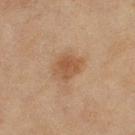Assessment:
The lesion was tiled from a total-body skin photograph and was not biopsied.
Clinical summary:
The patient is a female aged approximately 60. The lesion-visualizer software estimated a footprint of about 8 mm², an outline eccentricity of about 0.6 (0 = round, 1 = elongated), and a shape-asymmetry score of about 0.2 (0 = symmetric). It also reported an average lesion color of about L≈48 a*≈18 b*≈32 (CIELAB), roughly 8 lightness units darker than nearby skin, and a normalized lesion–skin contrast near 6.5. A region of skin cropped from a whole-body photographic capture, roughly 15 mm wide. Longest diameter approximately 3.5 mm. The tile uses cross-polarized illumination. On the right thigh.